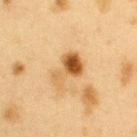A 15 mm crop from a total-body photograph taken for skin-cancer surveillance.
The subject is a female aged around 40.
The total-body-photography lesion software estimated a footprint of about 9.5 mm², an outline eccentricity of about 0.85 (0 = round, 1 = elongated), and a symmetry-axis asymmetry near 0.45. The analysis additionally found a mean CIELAB color near L≈49 a*≈18 b*≈37, roughly 12 lightness units darker than nearby skin, and a normalized border contrast of about 9.
The lesion is located on the left upper arm.
The recorded lesion diameter is about 4.5 mm.
The tile uses cross-polarized illumination.